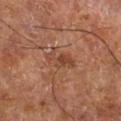• follow-up · catalogued during a skin exam; not biopsied
• location · the right lower leg
• size · ≈4 mm
• patient · aged approximately 65
• tile lighting · cross-polarized
• automated lesion analysis · an average lesion color of about L≈43 a*≈24 b*≈31 (CIELAB); a classifier nevus-likeness of about 25/100 and a detector confidence of about 100 out of 100 that the crop contains a lesion
• acquisition · total-body-photography crop, ~15 mm field of view